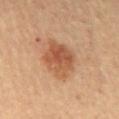No biopsy was performed on this lesion — it was imaged during a full skin examination and was not determined to be concerning. Cropped from a total-body skin-imaging series; the visible field is about 15 mm. The lesion's longest dimension is about 5 mm. The subject is a female in their 60s. Located on the mid back. An algorithmic analysis of the crop reported a lesion area of about 14 mm², an outline eccentricity of about 0.6 (0 = round, 1 = elongated), and two-axis asymmetry of about 0.2. And it measured an average lesion color of about L≈50 a*≈22 b*≈33 (CIELAB), a lesion–skin lightness drop of about 10, and a normalized lesion–skin contrast near 7.5. The analysis additionally found a border-irregularity index near 2.5/10, a color-variation rating of about 4.5/10, and radial color variation of about 1.5. Imaged with cross-polarized lighting.